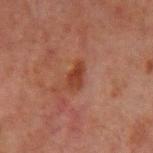follow-up=total-body-photography surveillance lesion; no biopsy | size=≈3 mm | patient=male, in their 60s | anatomic site=the left upper arm | image=~15 mm crop, total-body skin-cancer survey | lighting=cross-polarized illumination.